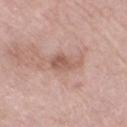Clinical impression:
This lesion was catalogued during total-body skin photography and was not selected for biopsy.
Acquisition and patient details:
Measured at roughly 4 mm in maximum diameter. Cropped from a whole-body photographic skin survey; the tile spans about 15 mm. The patient is a female about 70 years old. This is a white-light tile. An algorithmic analysis of the crop reported an average lesion color of about L≈57 a*≈20 b*≈26 (CIELAB), roughly 10 lightness units darker than nearby skin, and a normalized border contrast of about 6.5. The software also gave a detector confidence of about 100 out of 100 that the crop contains a lesion. The lesion is on the right thigh.